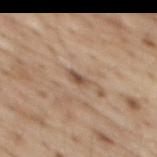<case>
<biopsy_status>not biopsied; imaged during a skin examination</biopsy_status>
<patient>
  <sex>male</sex>
  <age_approx>70</age_approx>
</patient>
<image>
  <source>total-body photography crop</source>
  <field_of_view_mm>15</field_of_view_mm>
</image>
<site>abdomen</site>
<lesion_size>
  <long_diameter_mm_approx>2.5</long_diameter_mm_approx>
</lesion_size>
</case>